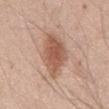follow-up — catalogued during a skin exam; not biopsied | lighting — white-light illumination | patient — male, aged 43–47 | body site — the abdomen | imaging modality — 15 mm crop, total-body photography | lesion diameter — ≈6 mm.